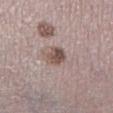A 15 mm close-up tile from a total-body photography series done for melanoma screening. From the left lower leg. The lesion's longest dimension is about 3 mm. The total-body-photography lesion software estimated a mean CIELAB color near L≈51 a*≈16 b*≈21, a lesion–skin lightness drop of about 12, and a lesion-to-skin contrast of about 8.5 (normalized; higher = more distinct). It also reported border irregularity of about 2 on a 0–10 scale, a color-variation rating of about 5.5/10, and radial color variation of about 2. It also reported a classifier nevus-likeness of about 60/100 and lesion-presence confidence of about 100/100. The patient is a male aged approximately 70.